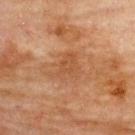Captured during whole-body skin photography for melanoma surveillance; the lesion was not biopsied. A female patient, aged 78–82. Longest diameter approximately 4 mm. The lesion is on the upper back. Cropped from a total-body skin-imaging series; the visible field is about 15 mm. Captured under cross-polarized illumination. The lesion-visualizer software estimated a lesion area of about 8.5 mm², a shape eccentricity near 0.7, and two-axis asymmetry of about 0.5. The analysis additionally found a color-variation rating of about 2.5/10 and a peripheral color-asymmetry measure near 1. It also reported lesion-presence confidence of about 100/100.